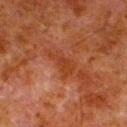Assessment:
The lesion was tiled from a total-body skin photograph and was not biopsied.
Context:
A male subject approximately 80 years of age. A close-up tile cropped from a whole-body skin photograph, about 15 mm across. The lesion is on the left lower leg.